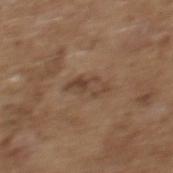Assessment: No biopsy was performed on this lesion — it was imaged during a full skin examination and was not determined to be concerning. Background: The lesion-visualizer software estimated an outline eccentricity of about 0.9 (0 = round, 1 = elongated) and a symmetry-axis asymmetry near 0.45. It also reported a mean CIELAB color near L≈43 a*≈17 b*≈27 and a normalized lesion–skin contrast near 6.5. And it measured a classifier nevus-likeness of about 0/100 and lesion-presence confidence of about 85/100. Approximately 4 mm at its widest. A 15 mm close-up extracted from a 3D total-body photography capture. The lesion is located on the mid back. The subject is a female approximately 65 years of age. Captured under white-light illumination.